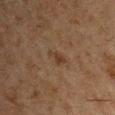Findings:
– follow-up: total-body-photography surveillance lesion; no biopsy
– patient: male, aged approximately 50
– location: the arm
– image source: total-body-photography crop, ~15 mm field of view